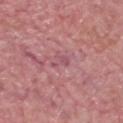subject: male, aged approximately 65 | size: ≈3 mm | tile lighting: white-light illumination | location: the head or neck | TBP lesion metrics: a lesion area of about 2.5 mm² and a shape-asymmetry score of about 0.5 (0 = symmetric); a mean CIELAB color near L≈52 a*≈28 b*≈16 and about 7 CIELAB-L* units darker than the surrounding skin; a border-irregularity index near 5.5/10; a classifier nevus-likeness of about 0/100 and a detector confidence of about 65 out of 100 that the crop contains a lesion | image source: 15 mm crop, total-body photography.